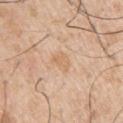The lesion was tiled from a total-body skin photograph and was not biopsied.